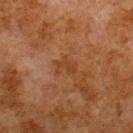The lesion was photographed on a routine skin check and not biopsied; there is no pathology result. The patient is a male roughly 80 years of age. The lesion is located on the upper back. A 15 mm close-up extracted from a 3D total-body photography capture.